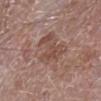This lesion was catalogued during total-body skin photography and was not selected for biopsy. Longest diameter approximately 5.5 mm. Imaged with white-light lighting. A male patient roughly 65 years of age. Located on the left lower leg. The lesion-visualizer software estimated lesion-presence confidence of about 100/100. This image is a 15 mm lesion crop taken from a total-body photograph.